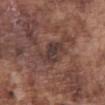{"biopsy_status": "not biopsied; imaged during a skin examination", "automated_metrics": {"area_mm2_approx": 7.0, "eccentricity": 0.85, "shape_asymmetry": 0.35, "color_variation_0_10": 3.5}, "lighting": "white-light", "site": "abdomen", "patient": {"sex": "male", "age_approx": 75}, "image": {"source": "total-body photography crop", "field_of_view_mm": 15}, "lesion_size": {"long_diameter_mm_approx": 4.0}}